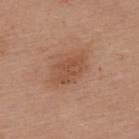Part of a total-body skin-imaging series; this lesion was reviewed on a skin check and was not flagged for biopsy. Located on the upper back. A 15 mm crop from a total-body photograph taken for skin-cancer surveillance. Automated image analysis of the tile measured a footprint of about 7 mm² and two-axis asymmetry of about 0.3. The analysis additionally found a mean CIELAB color near L≈50 a*≈22 b*≈33, roughly 7 lightness units darker than nearby skin, and a normalized border contrast of about 6. The software also gave a border-irregularity rating of about 3.5/10. And it measured a nevus-likeness score of about 25/100 and lesion-presence confidence of about 100/100. This is a white-light tile. The subject is a female in their 60s. The lesion's longest dimension is about 4 mm.